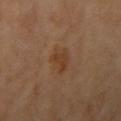workup = total-body-photography surveillance lesion; no biopsy | patient = female, roughly 65 years of age | image = ~15 mm crop, total-body skin-cancer survey | site = the left arm.